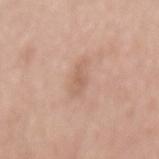{
  "biopsy_status": "not biopsied; imaged during a skin examination",
  "patient": {
    "sex": "female",
    "age_approx": 60
  },
  "image": {
    "source": "total-body photography crop",
    "field_of_view_mm": 15
  },
  "site": "mid back"
}